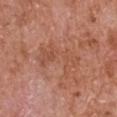notes: total-body-photography surveillance lesion; no biopsy.